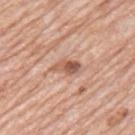Captured during whole-body skin photography for melanoma surveillance; the lesion was not biopsied. A male patient, in their 80s. A 15 mm crop from a total-body photograph taken for skin-cancer surveillance. The lesion is on the left upper arm.